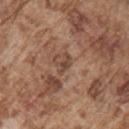From the right upper arm. Automated tile analysis of the lesion measured an area of roughly 3 mm², an eccentricity of roughly 0.85, and a symmetry-axis asymmetry near 0.35. The software also gave an automated nevus-likeness rating near 0 out of 100 and lesion-presence confidence of about 55/100. The tile uses white-light illumination. About 2.5 mm across. Cropped from a total-body skin-imaging series; the visible field is about 15 mm. A male patient, aged 73–77.